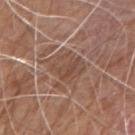biopsy_status: not biopsied; imaged during a skin examination
lighting: white-light
lesion_size:
  long_diameter_mm_approx: 4.5
image:
  source: total-body photography crop
  field_of_view_mm: 15
automated_metrics:
  cielab_L: 48
  cielab_a: 18
  cielab_b: 27
  vs_skin_darker_L: 7.0
  vs_skin_contrast_norm: 5.5
patient:
  sex: male
  age_approx: 80
site: right upper arm